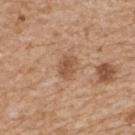notes: total-body-photography surveillance lesion; no biopsy
body site: the upper back
tile lighting: white-light
patient: female, roughly 70 years of age
lesion diameter: about 3 mm
TBP lesion metrics: an eccentricity of roughly 0.7 and a shape-asymmetry score of about 0.15 (0 = symmetric)
imaging modality: ~15 mm tile from a whole-body skin photo